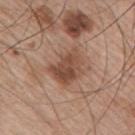Part of a total-body skin-imaging series; this lesion was reviewed on a skin check and was not flagged for biopsy. The tile uses white-light illumination. Automated tile analysis of the lesion measured an outline eccentricity of about 0.65 (0 = round, 1 = elongated) and a symmetry-axis asymmetry near 0.25. It also reported roughly 11 lightness units darker than nearby skin and a lesion-to-skin contrast of about 8 (normalized; higher = more distinct). And it measured an automated nevus-likeness rating near 20 out of 100 and a lesion-detection confidence of about 100/100. A 15 mm crop from a total-body photograph taken for skin-cancer surveillance. The patient is a male aged around 65. Located on the back.